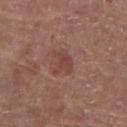<record>
<biopsy_status>not biopsied; imaged during a skin examination</biopsy_status>
<lesion_size>
  <long_diameter_mm_approx>2.5</long_diameter_mm_approx>
</lesion_size>
<image>
  <source>total-body photography crop</source>
  <field_of_view_mm>15</field_of_view_mm>
</image>
<lighting>white-light</lighting>
<automated_metrics>
  <cielab_L>43</cielab_L>
  <cielab_a>22</cielab_a>
  <cielab_b>24</cielab_b>
  <vs_skin_contrast_norm>6.0</vs_skin_contrast_norm>
</automated_metrics>
<site>right lower leg</site>
<patient>
  <sex>female</sex>
  <age_approx>80</age_approx>
</patient>
</record>